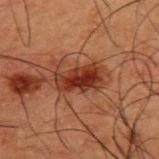| field | value |
|---|---|
| notes | total-body-photography surveillance lesion; no biopsy |
| image source | ~15 mm tile from a whole-body skin photo |
| subject | male, aged 48 to 52 |
| body site | the upper back |